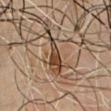biopsy status: catalogued during a skin exam; not biopsied | tile lighting: cross-polarized | size: ≈4.5 mm | subject: male, in their mid- to late 60s | image source: 15 mm crop, total-body photography | location: the chest.